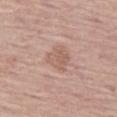Case summary:
- imaging modality · 15 mm crop, total-body photography
- patient · male, aged around 75
- location · the left thigh
- size · about 3.5 mm
- automated lesion analysis · an area of roughly 8 mm² and an outline eccentricity of about 0.55 (0 = round, 1 = elongated); an average lesion color of about L≈59 a*≈19 b*≈26 (CIELAB), about 7 CIELAB-L* units darker than the surrounding skin, and a lesion-to-skin contrast of about 5 (normalized; higher = more distinct); a border-irregularity index near 3/10, internal color variation of about 2.5 on a 0–10 scale, and peripheral color asymmetry of about 1; a classifier nevus-likeness of about 0/100 and a lesion-detection confidence of about 100/100